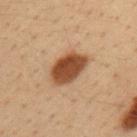Captured during whole-body skin photography for melanoma surveillance; the lesion was not biopsied.
A close-up tile cropped from a whole-body skin photograph, about 15 mm across.
Located on the left upper arm.
A male patient aged 33–37.
Longest diameter approximately 5.5 mm.